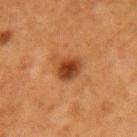biopsy_status: not biopsied; imaged during a skin examination
image:
  source: total-body photography crop
  field_of_view_mm: 15
automated_metrics:
  border_irregularity_0_10: 2.0
  color_variation_0_10: 5.0
  peripheral_color_asymmetry: 2.0
  lesion_detection_confidence_0_100: 100
site: arm
lighting: cross-polarized
patient:
  sex: female
  age_approx: 40